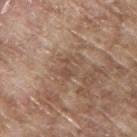Impression: No biopsy was performed on this lesion — it was imaged during a full skin examination and was not determined to be concerning. Context: From the upper back. The subject is a male aged approximately 65. The lesion's longest dimension is about 2.5 mm. A close-up tile cropped from a whole-body skin photograph, about 15 mm across.